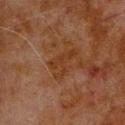notes: total-body-photography surveillance lesion; no biopsy | TBP lesion metrics: an area of roughly 6 mm², an eccentricity of roughly 0.85, and a shape-asymmetry score of about 0.65 (0 = symmetric); a within-lesion color-variation index near 0/10 and peripheral color asymmetry of about 0; a nevus-likeness score of about 0/100 | lighting: cross-polarized illumination | image source: ~15 mm crop, total-body skin-cancer survey | subject: male, roughly 80 years of age | site: the upper back.